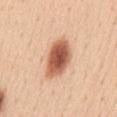workup = total-body-photography surveillance lesion; no biopsy
lesion size = about 5.5 mm
site = the chest
lighting = white-light illumination
acquisition = ~15 mm tile from a whole-body skin photo
patient = female, aged approximately 45